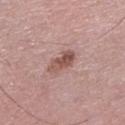Assessment:
This lesion was catalogued during total-body skin photography and was not selected for biopsy.
Clinical summary:
A 15 mm close-up extracted from a 3D total-body photography capture. A male subject aged 73–77. From the left lower leg. Longest diameter approximately 4 mm. The lesion-visualizer software estimated an average lesion color of about L≈52 a*≈21 b*≈23 (CIELAB). The software also gave a color-variation rating of about 5.5/10 and a peripheral color-asymmetry measure near 2.5. And it measured a nevus-likeness score of about 50/100 and lesion-presence confidence of about 100/100.